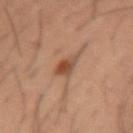Assessment: Captured during whole-body skin photography for melanoma surveillance; the lesion was not biopsied. Background: A roughly 15 mm field-of-view crop from a total-body skin photograph. A male subject, in their 40s. Longest diameter approximately 2.5 mm. From the right forearm.